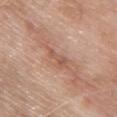Findings:
– illumination · white-light illumination
– automated metrics · a lesion color around L≈55 a*≈22 b*≈29 in CIELAB, roughly 8 lightness units darker than nearby skin, and a normalized border contrast of about 5.5; a border-irregularity index near 5/10, a color-variation rating of about 0.5/10, and peripheral color asymmetry of about 0; a classifier nevus-likeness of about 0/100 and a lesion-detection confidence of about 95/100
– lesion diameter · ≈3 mm
– subject · female, aged 73 to 77
– image · total-body-photography crop, ~15 mm field of view
– body site · the upper back